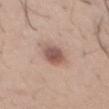Q: Was this lesion biopsied?
A: no biopsy performed (imaged during a skin exam)
Q: How was the tile lit?
A: white-light
Q: What is the lesion's diameter?
A: about 4.5 mm
Q: What is the anatomic site?
A: the abdomen
Q: Patient demographics?
A: male, aged approximately 30
Q: What kind of image is this?
A: total-body-photography crop, ~15 mm field of view